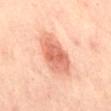The lesion was tiled from a total-body skin photograph and was not biopsied.